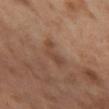Findings:
• follow-up · no biopsy performed (imaged during a skin exam)
• imaging modality · 15 mm crop, total-body photography
• size · about 3.5 mm
• patient · female, aged approximately 55
• automated metrics · a lesion area of about 5 mm² and an outline eccentricity of about 0.9 (0 = round, 1 = elongated); a mean CIELAB color near L≈45 a*≈19 b*≈29, about 7 CIELAB-L* units darker than the surrounding skin, and a normalized lesion–skin contrast near 6; a border-irregularity rating of about 5.5/10, a within-lesion color-variation index near 1/10, and radial color variation of about 0
• site · the left thigh